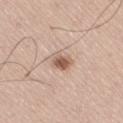No biopsy was performed on this lesion — it was imaged during a full skin examination and was not determined to be concerning. Imaged with white-light lighting. The total-body-photography lesion software estimated radial color variation of about 1. A close-up tile cropped from a whole-body skin photograph, about 15 mm across. The recorded lesion diameter is about 3 mm. The patient is a male about 60 years old. From the right thigh.